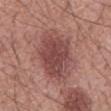Q: Was a biopsy performed?
A: total-body-photography surveillance lesion; no biopsy
Q: How large is the lesion?
A: ~7.5 mm (longest diameter)
Q: What are the patient's age and sex?
A: male, about 55 years old
Q: Where on the body is the lesion?
A: the mid back
Q: What is the imaging modality?
A: ~15 mm crop, total-body skin-cancer survey
Q: What did automated image analysis measure?
A: an area of roughly 25 mm², an outline eccentricity of about 0.85 (0 = round, 1 = elongated), and a symmetry-axis asymmetry near 0.15